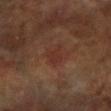Notes:
– workup · imaged on a skin check; not biopsied
– patient · female, aged 58 to 62
– imaging modality · ~15 mm tile from a whole-body skin photo
– TBP lesion metrics · a footprint of about 4 mm² and a shape-asymmetry score of about 0.45 (0 = symmetric); a border-irregularity rating of about 4.5/10, a color-variation rating of about 1.5/10, and a peripheral color-asymmetry measure near 0.5; a nevus-likeness score of about 25/100
– body site · the left forearm
– lighting · cross-polarized